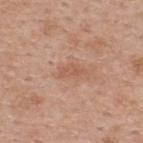The lesion was photographed on a routine skin check and not biopsied; there is no pathology result. The lesion's longest dimension is about 3 mm. The tile uses white-light illumination. A 15 mm close-up extracted from a 3D total-body photography capture. The subject is a male aged approximately 60. On the back. The total-body-photography lesion software estimated an eccentricity of roughly 0.9 and a symmetry-axis asymmetry near 0.4. The software also gave a mean CIELAB color near L≈57 a*≈22 b*≈32, roughly 7 lightness units darker than nearby skin, and a normalized lesion–skin contrast near 5.5.